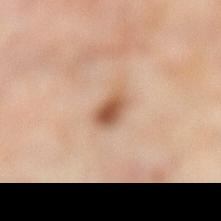Imaged during a routine full-body skin examination; the lesion was not biopsied and no histopathology is available. The lesion is located on the left lower leg. A female subject, aged 48–52. Cropped from a whole-body photographic skin survey; the tile spans about 15 mm.